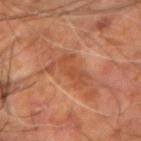Q: Was a biopsy performed?
A: no biopsy performed (imaged during a skin exam)
Q: Automated lesion metrics?
A: an eccentricity of roughly 0.85 and a symmetry-axis asymmetry near 0.45; internal color variation of about 2 on a 0–10 scale; an automated nevus-likeness rating near 0 out of 100 and lesion-presence confidence of about 80/100
Q: What are the patient's age and sex?
A: male, aged 58 to 62
Q: What is the lesion's diameter?
A: ≈4.5 mm
Q: Lesion location?
A: the left arm
Q: What is the imaging modality?
A: ~15 mm tile from a whole-body skin photo
Q: Illumination type?
A: cross-polarized